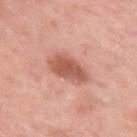follow-up — no biopsy performed (imaged during a skin exam)
patient — female, in their 50s
image source — ~15 mm tile from a whole-body skin photo
automated lesion analysis — a lesion area of about 10 mm²; a mean CIELAB color near L≈57 a*≈27 b*≈29, about 12 CIELAB-L* units darker than the surrounding skin, and a normalized lesion–skin contrast near 8; a border-irregularity index near 2.5/10 and internal color variation of about 3 on a 0–10 scale
tile lighting — white-light illumination
location — the right upper arm
size — ≈4 mm